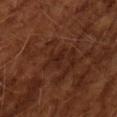Clinical impression: The lesion was photographed on a routine skin check and not biopsied; there is no pathology result. Clinical summary: This is a cross-polarized tile. The total-body-photography lesion software estimated an area of roughly 3 mm², an outline eccentricity of about 0.8 (0 = round, 1 = elongated), and a shape-asymmetry score of about 0.55 (0 = symmetric). The analysis additionally found a lesion color around L≈22 a*≈21 b*≈24 in CIELAB, about 5 CIELAB-L* units darker than the surrounding skin, and a lesion-to-skin contrast of about 5.5 (normalized; higher = more distinct). And it measured a border-irregularity rating of about 5.5/10 and a within-lesion color-variation index near 1/10. It also reported an automated nevus-likeness rating near 0 out of 100 and a detector confidence of about 95 out of 100 that the crop contains a lesion. A male patient aged around 65. About 3 mm across. A 15 mm crop from a total-body photograph taken for skin-cancer surveillance.